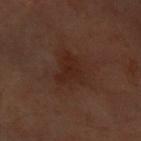This lesion was catalogued during total-body skin photography and was not selected for biopsy. Longest diameter approximately 3.5 mm. The lesion is located on the front of the torso. The lesion-visualizer software estimated a footprint of about 7 mm² and an outline eccentricity of about 0.7 (0 = round, 1 = elongated). And it measured a border-irregularity index near 4/10, internal color variation of about 1.5 on a 0–10 scale, and radial color variation of about 0.5. The analysis additionally found a nevus-likeness score of about 0/100 and lesion-presence confidence of about 100/100. A 15 mm close-up extracted from a 3D total-body photography capture. The subject is a female aged around 60. This is a cross-polarized tile.